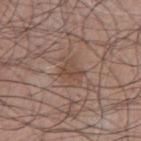Image and clinical context:
The recorded lesion diameter is about 2.5 mm. The subject is a male aged approximately 75. A 15 mm crop from a total-body photograph taken for skin-cancer surveillance. On the chest.